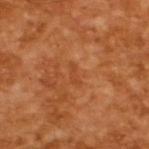No biopsy was performed on this lesion — it was imaged during a full skin examination and was not determined to be concerning. The lesion's longest dimension is about 2.5 mm. This is a cross-polarized tile. This image is a 15 mm lesion crop taken from a total-body photograph. A male subject, aged 63 to 67. The lesion-visualizer software estimated an average lesion color of about L≈45 a*≈28 b*≈40 (CIELAB) and roughly 6 lightness units darker than nearby skin. And it measured a border-irregularity rating of about 4/10, a within-lesion color-variation index near 0/10, and radial color variation of about 0. And it measured a classifier nevus-likeness of about 0/100 and lesion-presence confidence of about 100/100.